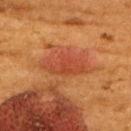The lesion was photographed on a routine skin check and not biopsied; there is no pathology result. The tile uses cross-polarized illumination. A 15 mm close-up extracted from a 3D total-body photography capture. The lesion-visualizer software estimated a lesion area of about 11 mm² and a shape-asymmetry score of about 0.2 (0 = symmetric). It also reported a lesion color around L≈39 a*≈27 b*≈33 in CIELAB, roughly 7 lightness units darker than nearby skin, and a normalized border contrast of about 6. It also reported a nevus-likeness score of about 90/100 and a detector confidence of about 100 out of 100 that the crop contains a lesion. A female subject aged approximately 50. Located on the upper back. The lesion's longest dimension is about 5.5 mm.